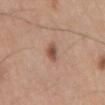{"biopsy_status": "not biopsied; imaged during a skin examination", "image": {"source": "total-body photography crop", "field_of_view_mm": 15}, "patient": {"sex": "male", "age_approx": 80}, "site": "mid back", "automated_metrics": {"area_mm2_approx": 4.0, "eccentricity": 0.8, "shape_asymmetry": 0.2, "border_irregularity_0_10": 2.0, "color_variation_0_10": 4.0, "peripheral_color_asymmetry": 1.5, "nevus_likeness_0_100": 95, "lesion_detection_confidence_0_100": 100}, "lesion_size": {"long_diameter_mm_approx": 3.0}}